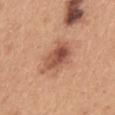Imaged during a routine full-body skin examination; the lesion was not biopsied and no histopathology is available. A female patient, about 30 years old. Located on the back. A 15 mm close-up tile from a total-body photography series done for melanoma screening.